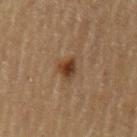illumination=cross-polarized illumination; body site=the left upper arm; acquisition=~15 mm tile from a whole-body skin photo; patient=male, in their 60s; size=about 2.5 mm.